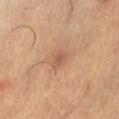Part of a total-body skin-imaging series; this lesion was reviewed on a skin check and was not flagged for biopsy. A female subject aged around 30. A 15 mm close-up extracted from a 3D total-body photography capture. Imaged with cross-polarized lighting. The lesion is on the abdomen.